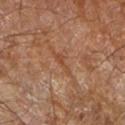Impression: Imaged during a routine full-body skin examination; the lesion was not biopsied and no histopathology is available. Background: Cropped from a whole-body photographic skin survey; the tile spans about 15 mm. A male subject aged 83 or older. The lesion is located on the right forearm.